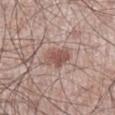<case>
  <biopsy_status>not biopsied; imaged during a skin examination</biopsy_status>
  <lesion_size>
    <long_diameter_mm_approx>3.0</long_diameter_mm_approx>
  </lesion_size>
  <site>right lower leg</site>
  <image>
    <source>total-body photography crop</source>
    <field_of_view_mm>15</field_of_view_mm>
  </image>
  <automated_metrics>
    <border_irregularity_0_10>2.5</border_irregularity_0_10>
    <color_variation_0_10>3.0</color_variation_0_10>
    <peripheral_color_asymmetry>1.0</peripheral_color_asymmetry>
    <nevus_likeness_0_100>95</nevus_likeness_0_100>
    <lesion_detection_confidence_0_100>100</lesion_detection_confidence_0_100>
  </automated_metrics>
  <patient>
    <sex>male</sex>
    <age_approx>55</age_approx>
  </patient>
</case>